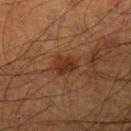Imaged during a routine full-body skin examination; the lesion was not biopsied and no histopathology is available.